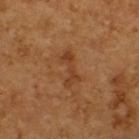Imaged with cross-polarized lighting. Located on the upper back. About 4.5 mm across. A male patient in their 60s. A roughly 15 mm field-of-view crop from a total-body skin photograph.